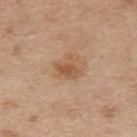This image is a 15 mm lesion crop taken from a total-body photograph.
A male subject, aged around 70.
Automated tile analysis of the lesion measured a lesion area of about 5.5 mm², an outline eccentricity of about 0.45 (0 = round, 1 = elongated), and a shape-asymmetry score of about 0.35 (0 = symmetric). The software also gave a mean CIELAB color near L≈55 a*≈19 b*≈34, roughly 9 lightness units darker than nearby skin, and a normalized lesion–skin contrast near 6.5.
The lesion is located on the back.
The recorded lesion diameter is about 2.5 mm.